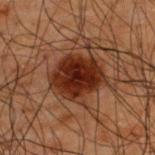Clinical impression: No biopsy was performed on this lesion — it was imaged during a full skin examination and was not determined to be concerning. Acquisition and patient details: The lesion's longest dimension is about 5 mm. A male patient roughly 50 years of age. A 15 mm close-up tile from a total-body photography series done for melanoma screening. The lesion is on the upper back. Automated image analysis of the tile measured a mean CIELAB color near L≈21 a*≈20 b*≈23. It also reported border irregularity of about 2 on a 0–10 scale, internal color variation of about 4.5 on a 0–10 scale, and peripheral color asymmetry of about 1. The software also gave a nevus-likeness score of about 100/100.